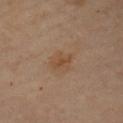<case>
<biopsy_status>not biopsied; imaged during a skin examination</biopsy_status>
<lighting>cross-polarized</lighting>
<site>left upper arm</site>
<image>
  <source>total-body photography crop</source>
  <field_of_view_mm>15</field_of_view_mm>
</image>
<patient>
  <sex>female</sex>
  <age_approx>60</age_approx>
</patient>
<lesion_size>
  <long_diameter_mm_approx>3.0</long_diameter_mm_approx>
</lesion_size>
</case>